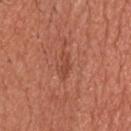Q: Was this lesion biopsied?
A: catalogued during a skin exam; not biopsied
Q: Lesion size?
A: ~2.5 mm (longest diameter)
Q: What is the anatomic site?
A: the head or neck
Q: What lighting was used for the tile?
A: white-light illumination
Q: Patient demographics?
A: male, aged approximately 55
Q: What kind of image is this?
A: ~15 mm tile from a whole-body skin photo
Q: What did automated image analysis measure?
A: a border-irregularity rating of about 4.5/10 and a within-lesion color-variation index near 0/10; a nevus-likeness score of about 0/100 and a lesion-detection confidence of about 100/100